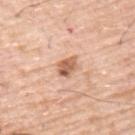{"lesion_size": {"long_diameter_mm_approx": 3.0}, "site": "upper back", "lighting": "white-light", "image": {"source": "total-body photography crop", "field_of_view_mm": 15}, "patient": {"sex": "male", "age_approx": 70}}